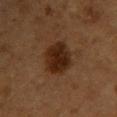Recorded during total-body skin imaging; not selected for excision or biopsy. This is a cross-polarized tile. A male patient aged approximately 55. Measured at roughly 4.5 mm in maximum diameter. A region of skin cropped from a whole-body photographic capture, roughly 15 mm wide. Automated tile analysis of the lesion measured a lesion color around L≈21 a*≈17 b*≈25 in CIELAB. The analysis additionally found a border-irregularity rating of about 1.5/10, internal color variation of about 3.5 on a 0–10 scale, and peripheral color asymmetry of about 1. The lesion is located on the upper back.